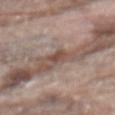Q: What is the anatomic site?
A: the mid back
Q: Patient demographics?
A: male, aged approximately 80
Q: What kind of image is this?
A: 15 mm crop, total-body photography
Q: Automated lesion metrics?
A: an area of roughly 3 mm², an eccentricity of roughly 0.8, and two-axis asymmetry of about 0.45; a lesion–skin lightness drop of about 9 and a lesion-to-skin contrast of about 7.5 (normalized; higher = more distinct); internal color variation of about 1 on a 0–10 scale and a peripheral color-asymmetry measure near 0.5; a classifier nevus-likeness of about 0/100 and a lesion-detection confidence of about 95/100